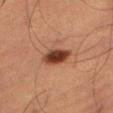The lesion was tiled from a total-body skin photograph and was not biopsied.
The lesion is on the right thigh.
A 15 mm crop from a total-body photograph taken for skin-cancer surveillance.
The subject is a male aged 73–77.